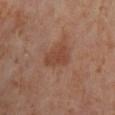{
  "biopsy_status": "not biopsied; imaged during a skin examination",
  "patient": {
    "sex": "female",
    "age_approx": 55
  },
  "lighting": "cross-polarized",
  "image": {
    "source": "total-body photography crop",
    "field_of_view_mm": 15
  },
  "site": "right lower leg"
}